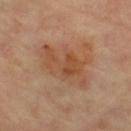Clinical impression: This lesion was catalogued during total-body skin photography and was not selected for biopsy. Context: An algorithmic analysis of the crop reported a lesion area of about 15 mm², an eccentricity of roughly 0.85, and two-axis asymmetry of about 0.45. And it measured a lesion–skin lightness drop of about 8 and a lesion-to-skin contrast of about 6.5 (normalized; higher = more distinct). A female subject, aged 68 to 72. Longest diameter approximately 6.5 mm. Located on the right lower leg. A close-up tile cropped from a whole-body skin photograph, about 15 mm across. This is a cross-polarized tile.